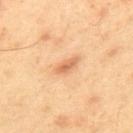An algorithmic analysis of the crop reported an outline eccentricity of about 0.9 (0 = round, 1 = elongated) and a shape-asymmetry score of about 0.25 (0 = symmetric).
A close-up tile cropped from a whole-body skin photograph, about 15 mm across.
A male patient in their mid-40s.
The lesion is on the back.
Approximately 3 mm at its widest.